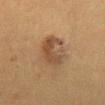Q: Was a biopsy performed?
A: catalogued during a skin exam; not biopsied
Q: Lesion size?
A: about 5 mm
Q: Lesion location?
A: the front of the torso
Q: What are the patient's age and sex?
A: female, aged 48–52
Q: How was the tile lit?
A: cross-polarized
Q: What is the imaging modality?
A: ~15 mm crop, total-body skin-cancer survey